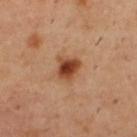Part of a total-body skin-imaging series; this lesion was reviewed on a skin check and was not flagged for biopsy.
Cropped from a total-body skin-imaging series; the visible field is about 15 mm.
Automated tile analysis of the lesion measured a shape-asymmetry score of about 0.2 (0 = symmetric). The software also gave an average lesion color of about L≈43 a*≈24 b*≈34 (CIELAB), roughly 14 lightness units darker than nearby skin, and a normalized lesion–skin contrast near 10.5. The analysis additionally found a nevus-likeness score of about 100/100.
The recorded lesion diameter is about 3 mm.
This is a cross-polarized tile.
On the back.
A male subject, aged approximately 55.